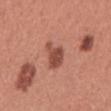Assessment: Part of a total-body skin-imaging series; this lesion was reviewed on a skin check and was not flagged for biopsy. Context: The total-body-photography lesion software estimated a mean CIELAB color near L≈49 a*≈27 b*≈29, roughly 12 lightness units darker than nearby skin, and a lesion-to-skin contrast of about 8.5 (normalized; higher = more distinct). And it measured a border-irregularity index near 3.5/10 and internal color variation of about 2.5 on a 0–10 scale. Located on the abdomen. A male patient, roughly 45 years of age. Cropped from a whole-body photographic skin survey; the tile spans about 15 mm. Captured under white-light illumination. Measured at roughly 4 mm in maximum diameter.